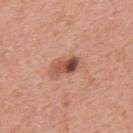Q: Was this lesion biopsied?
A: no biopsy performed (imaged during a skin exam)